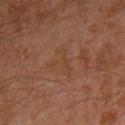| field | value |
|---|---|
| workup | imaged on a skin check; not biopsied |
| site | the arm |
| illumination | cross-polarized |
| acquisition | ~15 mm tile from a whole-body skin photo |
| patient | male, approximately 30 years of age |
| image-analysis metrics | a lesion–skin lightness drop of about 4 and a lesion-to-skin contrast of about 5 (normalized; higher = more distinct); a color-variation rating of about 1.5/10 and peripheral color asymmetry of about 0.5 |
| size | ~3.5 mm (longest diameter) |